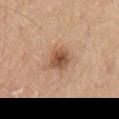Impression:
Imaged during a routine full-body skin examination; the lesion was not biopsied and no histopathology is available.
Clinical summary:
A 15 mm crop from a total-body photograph taken for skin-cancer surveillance. Automated image analysis of the tile measured a shape eccentricity near 0.6 and a symmetry-axis asymmetry near 0.2. It also reported a classifier nevus-likeness of about 90/100 and a lesion-detection confidence of about 100/100. Captured under white-light illumination. From the right upper arm. Longest diameter approximately 3.5 mm. A male patient, aged around 80.